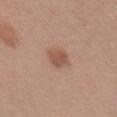The lesion was tiled from a total-body skin photograph and was not biopsied. A lesion tile, about 15 mm wide, cut from a 3D total-body photograph. The subject is a female aged around 35. On the head or neck. This is a white-light tile. Measured at roughly 2.5 mm in maximum diameter.